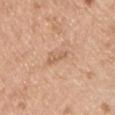Recorded during total-body skin imaging; not selected for excision or biopsy.
From the chest.
A male patient, roughly 60 years of age.
A 15 mm close-up tile from a total-body photography series done for melanoma screening.